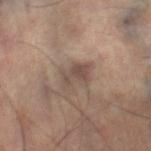Recorded during total-body skin imaging; not selected for excision or biopsy. Captured under cross-polarized illumination. About 3.5 mm across. The lesion is on the left leg. A roughly 15 mm field-of-view crop from a total-body skin photograph. The patient is a male aged approximately 50.